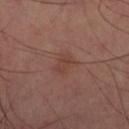notes — no biopsy performed (imaged during a skin exam) | image source — ~15 mm tile from a whole-body skin photo | anatomic site — the leg.